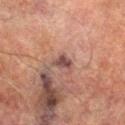Captured during whole-body skin photography for melanoma surveillance; the lesion was not biopsied. A male subject, aged approximately 75. A 15 mm close-up tile from a total-body photography series done for melanoma screening. Approximately 2.5 mm at its widest. The lesion-visualizer software estimated an area of roughly 3.5 mm², an eccentricity of roughly 0.75, and two-axis asymmetry of about 0.35. And it measured an average lesion color of about L≈39 a*≈18 b*≈19 (CIELAB) and a lesion–skin lightness drop of about 10. And it measured a classifier nevus-likeness of about 0/100 and a detector confidence of about 100 out of 100 that the crop contains a lesion. The lesion is located on the right lower leg. This is a cross-polarized tile.